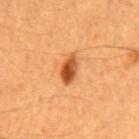Context: A male subject, approximately 60 years of age. A region of skin cropped from a whole-body photographic capture, roughly 15 mm wide. Located on the mid back.